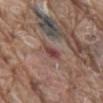Q: Is there a histopathology result?
A: total-body-photography surveillance lesion; no biopsy